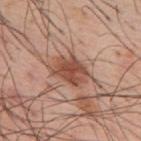Captured during whole-body skin photography for melanoma surveillance; the lesion was not biopsied. A close-up tile cropped from a whole-body skin photograph, about 15 mm across. On the back. About 4.5 mm across. An algorithmic analysis of the crop reported an average lesion color of about L≈50 a*≈23 b*≈29 (CIELAB) and a normalized lesion–skin contrast near 8.5. The software also gave border irregularity of about 3 on a 0–10 scale, internal color variation of about 4 on a 0–10 scale, and a peripheral color-asymmetry measure near 1. And it measured a classifier nevus-likeness of about 90/100 and a lesion-detection confidence of about 100/100. Captured under white-light illumination. A male subject about 55 years old.